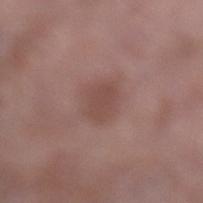Recorded during total-body skin imaging; not selected for excision or biopsy.
A region of skin cropped from a whole-body photographic capture, roughly 15 mm wide.
The lesion is located on the left lower leg.
This is a white-light tile.
A female patient aged around 70.